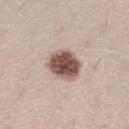workup = catalogued during a skin exam; not biopsied
subject = female, aged 38 to 42
size = about 4 mm
image source = ~15 mm crop, total-body skin-cancer survey
body site = the left lower leg